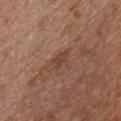Part of a total-body skin-imaging series; this lesion was reviewed on a skin check and was not flagged for biopsy.
A female patient, approximately 40 years of age.
Captured under white-light illumination.
A lesion tile, about 15 mm wide, cut from a 3D total-body photograph.
The recorded lesion diameter is about 2.5 mm.
An algorithmic analysis of the crop reported about 7 CIELAB-L* units darker than the surrounding skin and a lesion-to-skin contrast of about 6 (normalized; higher = more distinct).
From the chest.